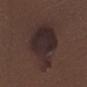The lesion was tiled from a total-body skin photograph and was not biopsied.
On the chest.
A female subject, approximately 5 years of age.
Cropped from a whole-body photographic skin survey; the tile spans about 15 mm.
The lesion-visualizer software estimated a lesion–skin lightness drop of about 8 and a normalized lesion–skin contrast near 10.5. And it measured a classifier nevus-likeness of about 45/100 and lesion-presence confidence of about 100/100.
The tile uses white-light illumination.